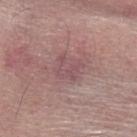biopsy status = no biopsy performed (imaged during a skin exam); lighting = white-light; location = the left forearm; acquisition = ~15 mm tile from a whole-body skin photo; lesion size = ≈3 mm; subject = female, aged around 65.